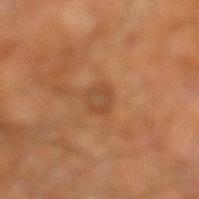The lesion was tiled from a total-body skin photograph and was not biopsied.
The recorded lesion diameter is about 2.5 mm.
A male subject aged 58–62.
The lesion is on the right leg.
Automated image analysis of the tile measured an average lesion color of about L≈46 a*≈21 b*≈34 (CIELAB). The software also gave an automated nevus-likeness rating near 0 out of 100 and a lesion-detection confidence of about 100/100.
A region of skin cropped from a whole-body photographic capture, roughly 15 mm wide.